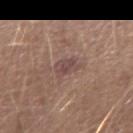Q: Lesion size?
A: ~2.5 mm (longest diameter)
Q: What is the imaging modality?
A: ~15 mm tile from a whole-body skin photo
Q: Where on the body is the lesion?
A: the left forearm
Q: What lighting was used for the tile?
A: white-light
Q: What did automated image analysis measure?
A: an average lesion color of about L≈46 a*≈18 b*≈20 (CIELAB), roughly 7 lightness units darker than nearby skin, and a normalized border contrast of about 6.5; an automated nevus-likeness rating near 0 out of 100 and lesion-presence confidence of about 100/100
Q: Patient demographics?
A: male, aged 23–27